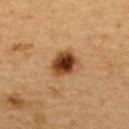{"biopsy_status": "not biopsied; imaged during a skin examination", "site": "upper back", "image": {"source": "total-body photography crop", "field_of_view_mm": 15}, "lighting": "cross-polarized", "automated_metrics": {"area_mm2_approx": 9.0, "eccentricity": 0.55, "shape_asymmetry": 0.2, "cielab_L": 45, "cielab_a": 23, "cielab_b": 37, "vs_skin_darker_L": 18.0, "border_irregularity_0_10": 2.0, "peripheral_color_asymmetry": 2.0}, "patient": {"sex": "female", "age_approx": 40}, "lesion_size": {"long_diameter_mm_approx": 3.5}}